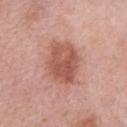Findings:
- workup — catalogued during a skin exam; not biopsied
- acquisition — 15 mm crop, total-body photography
- image-analysis metrics — an average lesion color of about L≈55 a*≈25 b*≈29 (CIELAB) and roughly 12 lightness units darker than nearby skin; border irregularity of about 2 on a 0–10 scale and a color-variation rating of about 4.5/10; lesion-presence confidence of about 100/100
- lighting — white-light
- body site — the right upper arm
- subject — female, roughly 65 years of age